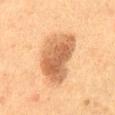* workup: total-body-photography surveillance lesion; no biopsy
* patient: female, aged 48 to 52
* body site: the front of the torso
* illumination: cross-polarized
* image: total-body-photography crop, ~15 mm field of view
* lesion diameter: about 7 mm
* image-analysis metrics: a mean CIELAB color near L≈51 a*≈19 b*≈33, a lesion–skin lightness drop of about 13, and a lesion-to-skin contrast of about 8.5 (normalized; higher = more distinct); a classifier nevus-likeness of about 80/100 and a detector confidence of about 100 out of 100 that the crop contains a lesion